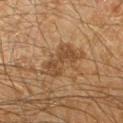{
  "biopsy_status": "not biopsied; imaged during a skin examination",
  "patient": {
    "sex": "male",
    "age_approx": 65
  },
  "site": "right forearm",
  "image": {
    "source": "total-body photography crop",
    "field_of_view_mm": 15
  },
  "lesion_size": {
    "long_diameter_mm_approx": 4.5
  },
  "automated_metrics": {
    "nevus_likeness_0_100": 5,
    "lesion_detection_confidence_0_100": 100
  },
  "lighting": "cross-polarized"
}